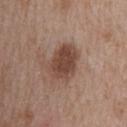<lesion>
  <biopsy_status>not biopsied; imaged during a skin examination</biopsy_status>
  <site>upper back</site>
  <image>
    <source>total-body photography crop</source>
    <field_of_view_mm>15</field_of_view_mm>
  </image>
  <patient>
    <sex>female</sex>
    <age_approx>45</age_approx>
  </patient>
  <lighting>white-light</lighting>
  <lesion_size>
    <long_diameter_mm_approx>5.0</long_diameter_mm_approx>
  </lesion_size>
  <automated_metrics>
    <area_mm2_approx>12.0</area_mm2_approx>
    <eccentricity>0.7</eccentricity>
    <shape_asymmetry>0.2</shape_asymmetry>
    <cielab_L>45</cielab_L>
    <cielab_a>19</cielab_a>
    <cielab_b>25</cielab_b>
    <nevus_likeness_0_100>85</nevus_likeness_0_100>
  </automated_metrics>
</lesion>